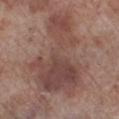follow-up = imaged on a skin check; not biopsied
location = the left lower leg
patient = male, aged 68 to 72
illumination = white-light illumination
lesion diameter = ~11 mm (longest diameter)
image = 15 mm crop, total-body photography